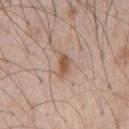Q: Was this lesion biopsied?
A: no biopsy performed (imaged during a skin exam)
Q: How large is the lesion?
A: ~2.5 mm (longest diameter)
Q: How was the tile lit?
A: white-light
Q: What kind of image is this?
A: 15 mm crop, total-body photography
Q: Lesion location?
A: the chest
Q: Patient demographics?
A: male, approximately 60 years of age